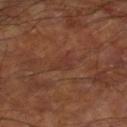<record>
  <biopsy_status>not biopsied; imaged during a skin examination</biopsy_status>
  <image>
    <source>total-body photography crop</source>
    <field_of_view_mm>15</field_of_view_mm>
  </image>
  <patient>
    <sex>male</sex>
    <age_approx>60</age_approx>
  </patient>
  <site>right lower leg</site>
</record>